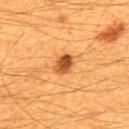No biopsy was performed on this lesion — it was imaged during a full skin examination and was not determined to be concerning. Automated tile analysis of the lesion measured a border-irregularity index near 2/10 and a color-variation rating of about 6.5/10. On the back. Approximately 2.5 mm at its widest. A 15 mm close-up tile from a total-body photography series done for melanoma screening. A male subject aged 58–62. Imaged with cross-polarized lighting.